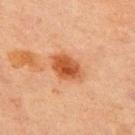Recorded during total-body skin imaging; not selected for excision or biopsy. The lesion-visualizer software estimated a lesion area of about 9 mm², an eccentricity of roughly 0.75, and a shape-asymmetry score of about 0.15 (0 = symmetric). And it measured a mean CIELAB color near L≈45 a*≈25 b*≈34 and a lesion–skin lightness drop of about 11. The analysis additionally found a border-irregularity index near 1.5/10, a color-variation rating of about 4.5/10, and peripheral color asymmetry of about 1.5. The subject is a male aged 68–72. On the chest. A 15 mm crop from a total-body photograph taken for skin-cancer surveillance.